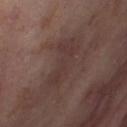Acquisition and patient details: A region of skin cropped from a whole-body photographic capture, roughly 15 mm wide. A female patient, aged 53–57. Located on the right thigh. Measured at roughly 6.5 mm in maximum diameter. Imaged with cross-polarized lighting.